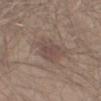Recorded during total-body skin imaging; not selected for excision or biopsy. Measured at roughly 5 mm in maximum diameter. A 15 mm close-up tile from a total-body photography series done for melanoma screening. This is a white-light tile. A male subject, about 45 years old. The lesion is on the right upper arm.